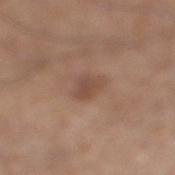The lesion was tiled from a total-body skin photograph and was not biopsied. A roughly 15 mm field-of-view crop from a total-body skin photograph. The lesion-visualizer software estimated roughly 8 lightness units darker than nearby skin and a normalized border contrast of about 6. The analysis additionally found a color-variation rating of about 1.5/10 and a peripheral color-asymmetry measure near 0.5. On the leg. The lesion's longest dimension is about 3 mm. The tile uses white-light illumination. A male patient, aged 63–67.